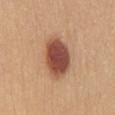The lesion was tiled from a total-body skin photograph and was not biopsied.